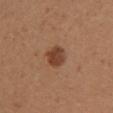| feature | finding |
|---|---|
| body site | the upper back |
| subject | female, aged 43 to 47 |
| image source | ~15 mm crop, total-body skin-cancer survey |
| lesion diameter | about 3 mm |
| image-analysis metrics | about 11 CIELAB-L* units darker than the surrounding skin and a normalized border contrast of about 9 |